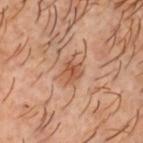Recorded during total-body skin imaging; not selected for excision or biopsy.
Measured at roughly 3.5 mm in maximum diameter.
The patient is a male about 50 years old.
A roughly 15 mm field-of-view crop from a total-body skin photograph.
The tile uses cross-polarized illumination.
The lesion is located on the head or neck.